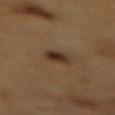notes: total-body-photography surveillance lesion; no biopsy | TBP lesion metrics: a lesion area of about 4.5 mm², a shape eccentricity near 0.6, and a symmetry-axis asymmetry near 0.2; an average lesion color of about L≈27 a*≈15 b*≈24 (CIELAB), about 9 CIELAB-L* units darker than the surrounding skin, and a normalized border contrast of about 10 | body site: the mid back | image: total-body-photography crop, ~15 mm field of view | patient: male, aged around 85 or older | lesion diameter: about 2.5 mm | illumination: cross-polarized illumination.